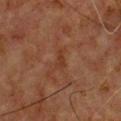workup: catalogued during a skin exam; not biopsied
anatomic site: the chest
size: ~3 mm (longest diameter)
image-analysis metrics: a lesion color around L≈30 a*≈19 b*≈27 in CIELAB and a lesion–skin lightness drop of about 5
image: 15 mm crop, total-body photography
lighting: cross-polarized illumination
subject: male, about 60 years old